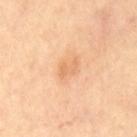Clinical impression: No biopsy was performed on this lesion — it was imaged during a full skin examination and was not determined to be concerning. Image and clinical context: Approximately 2.5 mm at its widest. Automated image analysis of the tile measured an area of roughly 3 mm² and a shape-asymmetry score of about 0.45 (0 = symmetric). The analysis additionally found a mean CIELAB color near L≈72 a*≈24 b*≈41, about 8 CIELAB-L* units darker than the surrounding skin, and a normalized lesion–skin contrast near 5. This is a cross-polarized tile. A lesion tile, about 15 mm wide, cut from a 3D total-body photograph. From the chest.